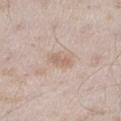biopsy status = no biopsy performed (imaged during a skin exam); location = the right thigh; lesion diameter = about 2.5 mm; image = total-body-photography crop, ~15 mm field of view; patient = male, in their mid-40s.